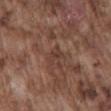Assessment:
Captured during whole-body skin photography for melanoma surveillance; the lesion was not biopsied.
Image and clinical context:
A male patient, aged 73 to 77. A close-up tile cropped from a whole-body skin photograph, about 15 mm across. An algorithmic analysis of the crop reported roughly 6 lightness units darker than nearby skin and a normalized border contrast of about 5.5. It also reported a border-irregularity index near 4.5/10, a color-variation rating of about 1.5/10, and peripheral color asymmetry of about 0.5. The lesion is located on the mid back. Approximately 2.5 mm at its widest.